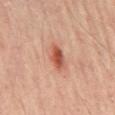notes: total-body-photography surveillance lesion; no biopsy | illumination: cross-polarized | acquisition: ~15 mm tile from a whole-body skin photo | patient: male, aged 63 to 67 | diameter: about 3 mm | site: the lower back | image-analysis metrics: border irregularity of about 2.5 on a 0–10 scale, a within-lesion color-variation index near 6/10, and radial color variation of about 2.